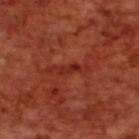Clinical impression:
This lesion was catalogued during total-body skin photography and was not selected for biopsy.
Clinical summary:
A male patient, approximately 70 years of age. The lesion is located on the upper back. This image is a 15 mm lesion crop taken from a total-body photograph. The tile uses cross-polarized illumination.